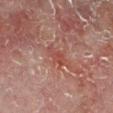Imaged during a routine full-body skin examination; the lesion was not biopsied and no histopathology is available. A male patient, in their 60s. The lesion-visualizer software estimated an eccentricity of roughly 0.85. The software also gave a lesion color around L≈42 a*≈25 b*≈24 in CIELAB, roughly 6 lightness units darker than nearby skin, and a normalized lesion–skin contrast near 5. A 15 mm crop from a total-body photograph taken for skin-cancer surveillance. Approximately 3 mm at its widest. On the left lower leg.